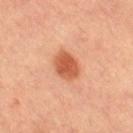notes = total-body-photography surveillance lesion; no biopsy | imaging modality = ~15 mm tile from a whole-body skin photo | patient = female, approximately 60 years of age | lighting = cross-polarized | image-analysis metrics = a symmetry-axis asymmetry near 0.15; a classifier nevus-likeness of about 100/100 and lesion-presence confidence of about 100/100 | site = the right thigh | size = ~4 mm (longest diameter).